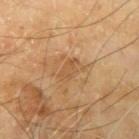Recorded during total-body skin imaging; not selected for excision or biopsy. A male patient approximately 65 years of age. A region of skin cropped from a whole-body photographic capture, roughly 15 mm wide. The lesion's longest dimension is about 2.5 mm. From the left upper arm. Imaged with cross-polarized lighting.